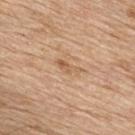| feature | finding |
|---|---|
| biopsy status | no biopsy performed (imaged during a skin exam) |
| subject | male, approximately 70 years of age |
| acquisition | ~15 mm crop, total-body skin-cancer survey |
| body site | the upper back |